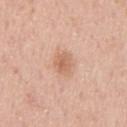Clinical impression:
This lesion was catalogued during total-body skin photography and was not selected for biopsy.
Clinical summary:
The subject is a male in their mid-50s. The lesion's longest dimension is about 2.5 mm. Located on the chest. A close-up tile cropped from a whole-body skin photograph, about 15 mm across. The total-body-photography lesion software estimated an average lesion color of about L≈63 a*≈22 b*≈32 (CIELAB), roughly 9 lightness units darker than nearby skin, and a normalized border contrast of about 6.5. The analysis additionally found a classifier nevus-likeness of about 50/100 and a detector confidence of about 100 out of 100 that the crop contains a lesion.